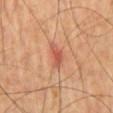{
  "image": {
    "source": "total-body photography crop",
    "field_of_view_mm": 15
  },
  "lesion_size": {
    "long_diameter_mm_approx": 3.5
  },
  "patient": {
    "sex": "male",
    "age_approx": 60
  },
  "automated_metrics": {
    "nevus_likeness_0_100": 25
  },
  "site": "mid back",
  "lighting": "cross-polarized"
}